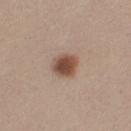Assessment:
This lesion was catalogued during total-body skin photography and was not selected for biopsy.
Context:
Located on the left thigh. The subject is a female aged 23 to 27. Approximately 3 mm at its widest. A region of skin cropped from a whole-body photographic capture, roughly 15 mm wide. This is a white-light tile.